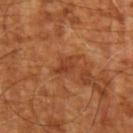biopsy_status: not biopsied; imaged during a skin examination
site: left upper arm
lesion_size:
  long_diameter_mm_approx: 3.0
image:
  source: total-body photography crop
  field_of_view_mm: 15
lighting: cross-polarized
automated_metrics:
  border_irregularity_0_10: 3.0
  color_variation_0_10: 3.0
  peripheral_color_asymmetry: 1.0
patient:
  age_approx: 65